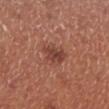workup = imaged on a skin check; not biopsied
lesion diameter = ~3 mm (longest diameter)
automated lesion analysis = a shape eccentricity near 0.65 and a symmetry-axis asymmetry near 0.25; a lesion color around L≈42 a*≈25 b*≈27 in CIELAB; a border-irregularity index near 3/10 and a within-lesion color-variation index near 3/10; a classifier nevus-likeness of about 65/100
subject = male, about 70 years old
image source = total-body-photography crop, ~15 mm field of view
illumination = white-light illumination
body site = the left lower leg